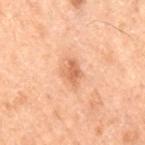Notes:
• follow-up · total-body-photography surveillance lesion; no biopsy